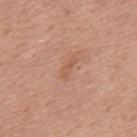Clinical impression:
This lesion was catalogued during total-body skin photography and was not selected for biopsy.
Context:
Imaged with white-light lighting. Longest diameter approximately 3 mm. A male subject aged 48–52. Located on the mid back. A 15 mm close-up tile from a total-body photography series done for melanoma screening.